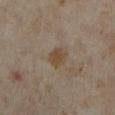notes=catalogued during a skin exam; not biopsied
subject=female, roughly 35 years of age
image=total-body-photography crop, ~15 mm field of view
site=the leg
size=≈3 mm